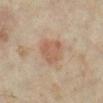biopsy status=total-body-photography surveillance lesion; no biopsy | patient=female, in their mid-30s | location=the left lower leg | image source=~15 mm crop, total-body skin-cancer survey.